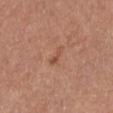Recorded during total-body skin imaging; not selected for excision or biopsy. The tile uses white-light illumination. The total-body-photography lesion software estimated a lesion area of about 2.5 mm² and an eccentricity of roughly 0.95. It also reported an automated nevus-likeness rating near 0 out of 100 and a lesion-detection confidence of about 100/100. A 15 mm close-up tile from a total-body photography series done for melanoma screening. Approximately 3 mm at its widest. The lesion is located on the chest. A female subject, aged approximately 45.